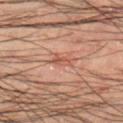workup = no biopsy performed (imaged during a skin exam)
lighting = cross-polarized illumination
subject = male, approximately 50 years of age
image = total-body-photography crop, ~15 mm field of view
lesion size = ~2.5 mm (longest diameter)
anatomic site = the right lower leg